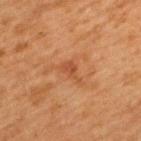Recorded during total-body skin imaging; not selected for excision or biopsy.
Cropped from a whole-body photographic skin survey; the tile spans about 15 mm.
The lesion is located on the upper back.
A female patient aged approximately 40.
The lesion-visualizer software estimated roughly 7 lightness units darker than nearby skin. The analysis additionally found a border-irregularity index near 5/10, internal color variation of about 1 on a 0–10 scale, and peripheral color asymmetry of about 0.5. And it measured an automated nevus-likeness rating near 0 out of 100.
Imaged with cross-polarized lighting.
The recorded lesion diameter is about 3 mm.